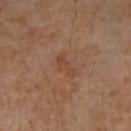biopsy status = total-body-photography surveillance lesion; no biopsy
lighting = cross-polarized illumination
anatomic site = the right upper arm
patient = female, approximately 45 years of age
acquisition = 15 mm crop, total-body photography
image-analysis metrics = a nevus-likeness score of about 0/100 and a lesion-detection confidence of about 100/100